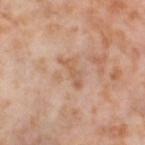The lesion was photographed on a routine skin check and not biopsied; there is no pathology result. Longest diameter approximately 4 mm. A roughly 15 mm field-of-view crop from a total-body skin photograph. From the left thigh. A female patient, aged 53–57.